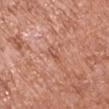<case>
  <biopsy_status>not biopsied; imaged during a skin examination</biopsy_status>
  <image>
    <source>total-body photography crop</source>
    <field_of_view_mm>15</field_of_view_mm>
  </image>
  <lighting>white-light</lighting>
  <site>left upper arm</site>
  <lesion_size>
    <long_diameter_mm_approx>2.5</long_diameter_mm_approx>
  </lesion_size>
  <patient>
    <sex>male</sex>
    <age_approx>65</age_approx>
  </patient>
</case>